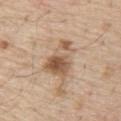Recorded during total-body skin imaging; not selected for excision or biopsy. On the abdomen. Cropped from a whole-body photographic skin survey; the tile spans about 15 mm. The total-body-photography lesion software estimated a lesion area of about 12 mm², an eccentricity of roughly 0.75, and a shape-asymmetry score of about 0.35 (0 = symmetric). This is a white-light tile. Approximately 5 mm at its widest. A male subject approximately 70 years of age.